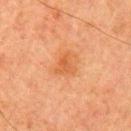biopsy status: imaged on a skin check; not biopsied | illumination: cross-polarized | diameter: ≈3 mm | image: ~15 mm tile from a whole-body skin photo | anatomic site: the left upper arm | subject: male, aged 78–82.